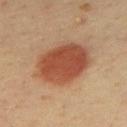{"biopsy_status": "not biopsied; imaged during a skin examination", "lighting": "cross-polarized", "patient": {"sex": "male", "age_approx": 40}, "site": "mid back", "lesion_size": {"long_diameter_mm_approx": 7.0}, "automated_metrics": {"area_mm2_approx": 25.0, "eccentricity": 0.75, "shape_asymmetry": 0.1, "cielab_L": 43, "cielab_a": 23, "cielab_b": 30, "vs_skin_darker_L": 12.0, "vs_skin_contrast_norm": 10.0}, "image": {"source": "total-body photography crop", "field_of_view_mm": 15}}